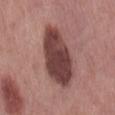follow-up = catalogued during a skin exam; not biopsied
patient = male, aged approximately 60
illumination = white-light
anatomic site = the back
acquisition = ~15 mm crop, total-body skin-cancer survey